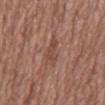site = the back | subject = female, in their mid-70s | size = ~4 mm (longest diameter) | imaging modality = ~15 mm crop, total-body skin-cancer survey | lighting = white-light.